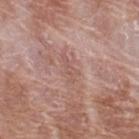Notes:
- biopsy status — catalogued during a skin exam; not biopsied
- size — about 3.5 mm
- lighting — white-light
- subject — female, aged approximately 60
- acquisition — total-body-photography crop, ~15 mm field of view
- automated metrics — a shape eccentricity near 0.95 and two-axis asymmetry of about 0.4; a border-irregularity rating of about 5.5/10, a within-lesion color-variation index near 0.5/10, and peripheral color asymmetry of about 0; an automated nevus-likeness rating near 0 out of 100 and a lesion-detection confidence of about 55/100
- body site — the upper back